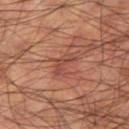follow-up: catalogued during a skin exam; not biopsied
imaging modality: total-body-photography crop, ~15 mm field of view
site: the right thigh
diameter: ~3.5 mm (longest diameter)
patient: male, in their 60s
automated metrics: a mean CIELAB color near L≈45 a*≈25 b*≈28, roughly 7 lightness units darker than nearby skin, and a lesion-to-skin contrast of about 5.5 (normalized; higher = more distinct); a border-irregularity rating of about 5.5/10, internal color variation of about 2 on a 0–10 scale, and peripheral color asymmetry of about 0.5